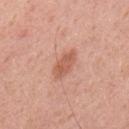{"biopsy_status": "not biopsied; imaged during a skin examination", "patient": {"sex": "male", "age_approx": 55}, "automated_metrics": {"cielab_L": 59, "cielab_a": 25, "cielab_b": 31, "vs_skin_contrast_norm": 6.5, "nevus_likeness_0_100": 55, "lesion_detection_confidence_0_100": 100}, "image": {"source": "total-body photography crop", "field_of_view_mm": 15}, "lesion_size": {"long_diameter_mm_approx": 4.0}, "site": "upper back"}